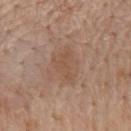Impression:
This lesion was catalogued during total-body skin photography and was not selected for biopsy.
Clinical summary:
A male patient aged around 60. The lesion is on the back. A close-up tile cropped from a whole-body skin photograph, about 15 mm across. An algorithmic analysis of the crop reported border irregularity of about 5 on a 0–10 scale, a color-variation rating of about 2/10, and a peripheral color-asymmetry measure near 0.5. It also reported a nevus-likeness score of about 0/100 and a lesion-detection confidence of about 100/100. Approximately 4.5 mm at its widest. Captured under white-light illumination.